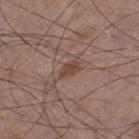| key | value |
|---|---|
| biopsy status | total-body-photography surveillance lesion; no biopsy |
| imaging modality | total-body-photography crop, ~15 mm field of view |
| lighting | white-light illumination |
| TBP lesion metrics | an average lesion color of about L≈43 a*≈19 b*≈24 (CIELAB) and a normalized border contrast of about 7.5; border irregularity of about 3 on a 0–10 scale and a within-lesion color-variation index near 1/10 |
| location | the left thigh |
| subject | male, aged 58–62 |
| size | about 2.5 mm |